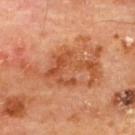The lesion was photographed on a routine skin check and not biopsied; there is no pathology result. From the upper back. A male patient, approximately 65 years of age. A roughly 15 mm field-of-view crop from a total-body skin photograph.